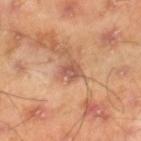Impression: The lesion was photographed on a routine skin check and not biopsied; there is no pathology result. Context: A 15 mm close-up tile from a total-body photography series done for melanoma screening. Located on the right lower leg. A male patient in their mid-40s.